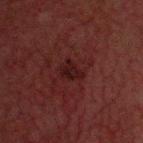lighting — cross-polarized illumination; size — about 3 mm; location — the head or neck; image — 15 mm crop, total-body photography; patient — male, about 60 years old.